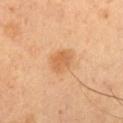Clinical impression: Part of a total-body skin-imaging series; this lesion was reviewed on a skin check and was not flagged for biopsy. Clinical summary: A male subject, in their 50s. Captured under cross-polarized illumination. The lesion is on the left thigh. A lesion tile, about 15 mm wide, cut from a 3D total-body photograph.